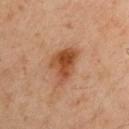Recorded during total-body skin imaging; not selected for excision or biopsy. The lesion-visualizer software estimated a footprint of about 11 mm², an outline eccentricity of about 0.7 (0 = round, 1 = elongated), and a shape-asymmetry score of about 0.35 (0 = symmetric). It also reported a lesion color around L≈48 a*≈25 b*≈36 in CIELAB, roughly 12 lightness units darker than nearby skin, and a lesion-to-skin contrast of about 9.5 (normalized; higher = more distinct). It also reported a border-irregularity rating of about 3.5/10, internal color variation of about 6 on a 0–10 scale, and a peripheral color-asymmetry measure near 2. The subject is a male approximately 40 years of age. A lesion tile, about 15 mm wide, cut from a 3D total-body photograph. The lesion is located on the left upper arm. The lesion's longest dimension is about 5 mm. Captured under cross-polarized illumination.